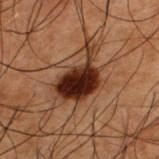Q: Was a biopsy performed?
A: imaged on a skin check; not biopsied
Q: Patient demographics?
A: male, roughly 50 years of age
Q: Automated lesion metrics?
A: a shape eccentricity near 0.8 and a shape-asymmetry score of about 0.45 (0 = symmetric)
Q: Illumination type?
A: cross-polarized illumination
Q: Lesion location?
A: the upper back
Q: How was this image acquired?
A: 15 mm crop, total-body photography
Q: What is the lesion's diameter?
A: ≈6.5 mm